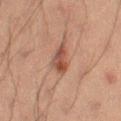{
  "biopsy_status": "not biopsied; imaged during a skin examination",
  "lighting": "cross-polarized",
  "site": "left thigh",
  "automated_metrics": {
    "area_mm2_approx": 5.5,
    "eccentricity": 0.85,
    "shape_asymmetry": 0.3,
    "peripheral_color_asymmetry": 2.5,
    "nevus_likeness_0_100": 85
  },
  "image": {
    "source": "total-body photography crop",
    "field_of_view_mm": 15
  },
  "patient": {
    "sex": "male",
    "age_approx": 55
  },
  "lesion_size": {
    "long_diameter_mm_approx": 4.0
  }
}